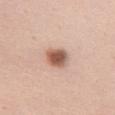This lesion was catalogued during total-body skin photography and was not selected for biopsy.
A female subject aged approximately 50.
From the mid back.
Cropped from a whole-body photographic skin survey; the tile spans about 15 mm.